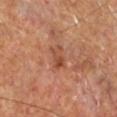Assessment:
Recorded during total-body skin imaging; not selected for excision or biopsy.
Acquisition and patient details:
On the right lower leg. About 2.5 mm across. The total-body-photography lesion software estimated an average lesion color of about L≈48 a*≈26 b*≈33 (CIELAB) and roughly 9 lightness units darker than nearby skin. It also reported border irregularity of about 4.5 on a 0–10 scale and peripheral color asymmetry of about 0.5. It also reported a classifier nevus-likeness of about 0/100 and a lesion-detection confidence of about 100/100. The tile uses cross-polarized illumination. A roughly 15 mm field-of-view crop from a total-body skin photograph. The patient is a male aged 63–67.